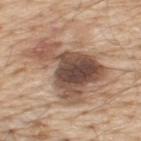Clinical impression:
No biopsy was performed on this lesion — it was imaged during a full skin examination and was not determined to be concerning.
Acquisition and patient details:
A male subject, roughly 70 years of age. Approximately 8.5 mm at its widest. The tile uses white-light illumination. Located on the upper back. A close-up tile cropped from a whole-body skin photograph, about 15 mm across. The total-body-photography lesion software estimated a lesion area of about 39 mm² and two-axis asymmetry of about 0.5. And it measured an average lesion color of about L≈52 a*≈17 b*≈27 (CIELAB) and a normalized border contrast of about 10. The analysis additionally found internal color variation of about 9.5 on a 0–10 scale and radial color variation of about 3. The software also gave a classifier nevus-likeness of about 15/100 and a detector confidence of about 100 out of 100 that the crop contains a lesion.